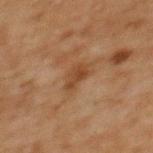notes = no biopsy performed (imaged during a skin exam); site = the back; subject = female, aged 58 to 62; image source = ~15 mm tile from a whole-body skin photo; lesion diameter = ≈3 mm; tile lighting = cross-polarized.